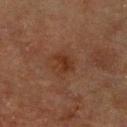notes: no biopsy performed (imaged during a skin exam) | image: 15 mm crop, total-body photography | size: about 3 mm | patient: male, aged approximately 70 | tile lighting: cross-polarized | location: the chest | automated metrics: a footprint of about 5.5 mm², a shape eccentricity near 0.65, and a shape-asymmetry score of about 0.3 (0 = symmetric); a mean CIELAB color near L≈27 a*≈18 b*≈25 and a normalized border contrast of about 6.5; a border-irregularity index near 3/10, a within-lesion color-variation index near 2.5/10, and radial color variation of about 1; a nevus-likeness score of about 15/100 and a lesion-detection confidence of about 100/100.